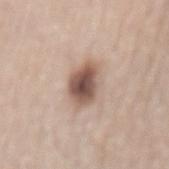Recorded during total-body skin imaging; not selected for excision or biopsy. Cropped from a whole-body photographic skin survey; the tile spans about 15 mm. The tile uses white-light illumination. The patient is a female aged approximately 60. On the mid back.